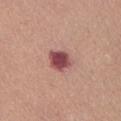follow-up = imaged on a skin check; not biopsied
anatomic site = the abdomen
subject = female, about 50 years old
imaging modality = ~15 mm crop, total-body skin-cancer survey
diameter = ≈3 mm
lighting = white-light